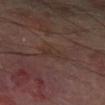Captured during whole-body skin photography for melanoma surveillance; the lesion was not biopsied.
A male patient, about 70 years old.
An algorithmic analysis of the crop reported an area of roughly 4 mm² and an eccentricity of roughly 0.8. The software also gave a lesion color around L≈30 a*≈15 b*≈20 in CIELAB and a lesion-to-skin contrast of about 4.5 (normalized; higher = more distinct). And it measured internal color variation of about 2.5 on a 0–10 scale and peripheral color asymmetry of about 1.
Measured at roughly 3 mm in maximum diameter.
A roughly 15 mm field-of-view crop from a total-body skin photograph.
The lesion is located on the left lower leg.